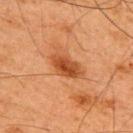This lesion was catalogued during total-body skin photography and was not selected for biopsy. Captured under cross-polarized illumination. The lesion-visualizer software estimated an eccentricity of roughly 0.85 and a shape-asymmetry score of about 0.2 (0 = symmetric). And it measured a mean CIELAB color near L≈40 a*≈25 b*≈36 and roughly 11 lightness units darker than nearby skin. A region of skin cropped from a whole-body photographic capture, roughly 15 mm wide. On the upper back. About 4 mm across. A male patient, in their mid-60s.